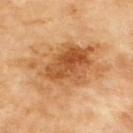{
  "biopsy_status": "not biopsied; imaged during a skin examination",
  "image": {
    "source": "total-body photography crop",
    "field_of_view_mm": 15
  },
  "lesion_size": {
    "long_diameter_mm_approx": 9.0
  },
  "site": "upper back"
}